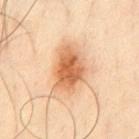{
  "biopsy_status": "not biopsied; imaged during a skin examination",
  "patient": {
    "sex": "male",
    "age_approx": 65
  },
  "lesion_size": {
    "long_diameter_mm_approx": 5.5
  },
  "image": {
    "source": "total-body photography crop",
    "field_of_view_mm": 15
  },
  "site": "chest",
  "lighting": "cross-polarized"
}